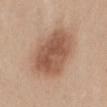Part of a total-body skin-imaging series; this lesion was reviewed on a skin check and was not flagged for biopsy.
Measured at roughly 7 mm in maximum diameter.
A female subject in their mid- to late 40s.
This image is a 15 mm lesion crop taken from a total-body photograph.
The tile uses white-light illumination.
The lesion is located on the mid back.
The lesion-visualizer software estimated roughly 12 lightness units darker than nearby skin and a normalized border contrast of about 8.5. And it measured a border-irregularity index near 1.5/10, internal color variation of about 4.5 on a 0–10 scale, and peripheral color asymmetry of about 1.5. The software also gave an automated nevus-likeness rating near 90 out of 100.